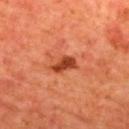{"biopsy_status": "not biopsied; imaged during a skin examination", "image": {"source": "total-body photography crop", "field_of_view_mm": 15}, "site": "mid back", "patient": {"sex": "male", "age_approx": 65}}